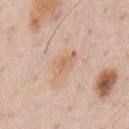A male patient about 60 years old. Located on the chest. A 15 mm crop from a total-body photograph taken for skin-cancer surveillance.